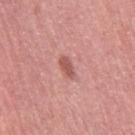No biopsy was performed on this lesion — it was imaged during a full skin examination and was not determined to be concerning. Located on the right thigh. A female patient aged 53–57. A roughly 15 mm field-of-view crop from a total-body skin photograph. Approximately 2.5 mm at its widest. Imaged with white-light lighting.